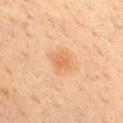The lesion was photographed on a routine skin check and not biopsied; there is no pathology result. A female patient, aged 53–57. A 15 mm crop from a total-body photograph taken for skin-cancer surveillance. Longest diameter approximately 3 mm. On the chest.